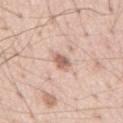The lesion was tiled from a total-body skin photograph and was not biopsied. The patient is a male aged 53 to 57. Cropped from a whole-body photographic skin survey; the tile spans about 15 mm. The tile uses white-light illumination. The lesion is on the chest. The total-body-photography lesion software estimated a lesion area of about 3.5 mm², a shape eccentricity near 0.8, and two-axis asymmetry of about 0.2. The analysis additionally found a lesion–skin lightness drop of about 13. And it measured a border-irregularity index near 2/10 and internal color variation of about 1.5 on a 0–10 scale. It also reported a classifier nevus-likeness of about 70/100 and lesion-presence confidence of about 100/100. The recorded lesion diameter is about 3 mm.